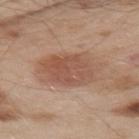Impression: Imaged during a routine full-body skin examination; the lesion was not biopsied and no histopathology is available. Background: A region of skin cropped from a whole-body photographic capture, roughly 15 mm wide. On the upper back. The patient is a male about 55 years old. The lesion's longest dimension is about 7.5 mm. The tile uses white-light illumination.